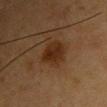Clinical impression:
The lesion was tiled from a total-body skin photograph and was not biopsied.
Image and clinical context:
An algorithmic analysis of the crop reported a lesion area of about 9 mm², an outline eccentricity of about 0.5 (0 = round, 1 = elongated), and a shape-asymmetry score of about 0.2 (0 = symmetric). The analysis additionally found a border-irregularity rating of about 2/10 and internal color variation of about 3 on a 0–10 scale. The lesion is located on the chest. Measured at roughly 3.5 mm in maximum diameter. Imaged with cross-polarized lighting. A female subject, approximately 45 years of age. Cropped from a whole-body photographic skin survey; the tile spans about 15 mm.